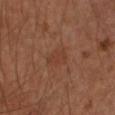The patient is a male in their mid- to late 40s. A 15 mm crop from a total-body photograph taken for skin-cancer surveillance. Located on the left forearm.